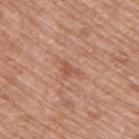subject = male, roughly 70 years of age | lesion diameter = ≈2.5 mm | lighting = white-light | body site = the upper back | automated metrics = a lesion area of about 2.5 mm² and a shape eccentricity near 0.8; a within-lesion color-variation index near 0.5/10 and radial color variation of about 0; a classifier nevus-likeness of about 0/100 and a detector confidence of about 100 out of 100 that the crop contains a lesion | image = ~15 mm crop, total-body skin-cancer survey.